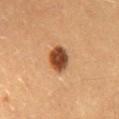follow-up=total-body-photography surveillance lesion; no biopsy
automated metrics=a nevus-likeness score of about 100/100 and a detector confidence of about 100 out of 100 that the crop contains a lesion
subject=male, aged approximately 20
size=~3.5 mm (longest diameter)
site=the lower back
illumination=cross-polarized
image source=total-body-photography crop, ~15 mm field of view